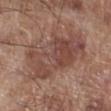notes = no biopsy performed (imaged during a skin exam); patient = male, aged around 70; location = the right lower leg; image-analysis metrics = a mean CIELAB color near L≈46 a*≈20 b*≈24 and a lesion–skin lightness drop of about 9; diameter = ≈8.5 mm; tile lighting = white-light; imaging modality = ~15 mm crop, total-body skin-cancer survey.